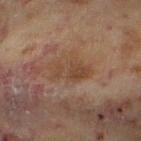Recorded during total-body skin imaging; not selected for excision or biopsy. The lesion is on the leg. A female patient, aged approximately 60. The recorded lesion diameter is about 5 mm. Captured under cross-polarized illumination. A roughly 15 mm field-of-view crop from a total-body skin photograph.